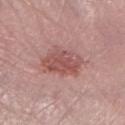Captured during whole-body skin photography for melanoma surveillance; the lesion was not biopsied.
The lesion is on the left lower leg.
A region of skin cropped from a whole-body photographic capture, roughly 15 mm wide.
A female subject, in their mid- to late 60s.
The recorded lesion diameter is about 5 mm.